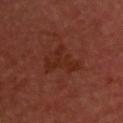Findings:
– biopsy status · no biopsy performed (imaged during a skin exam)
– image source · total-body-photography crop, ~15 mm field of view
– site · the upper back
– patient · male, approximately 50 years of age
– tile lighting · cross-polarized
– lesion size · ≈5 mm
– image-analysis metrics · internal color variation of about 2 on a 0–10 scale and peripheral color asymmetry of about 0.5; a classifier nevus-likeness of about 0/100 and a detector confidence of about 100 out of 100 that the crop contains a lesion